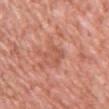Impression:
The lesion was photographed on a routine skin check and not biopsied; there is no pathology result.
Background:
A female subject, in their 40s. This is a white-light tile. Approximately 3 mm at its widest. The lesion is on the front of the torso. Automated image analysis of the tile measured an area of roughly 5 mm², an eccentricity of roughly 0.75, and two-axis asymmetry of about 0.3. And it measured a mean CIELAB color near L≈56 a*≈27 b*≈32 and a lesion-to-skin contrast of about 5 (normalized; higher = more distinct). And it measured a border-irregularity index near 4/10 and a color-variation rating of about 2.5/10. A 15 mm crop from a total-body photograph taken for skin-cancer surveillance.